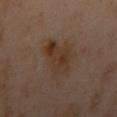  lighting: cross-polarized
  image:
    source: total-body photography crop
    field_of_view_mm: 15
  site: left arm
  patient:
    sex: female
    age_approx: 40
  lesion_size:
    long_diameter_mm_approx: 5.5